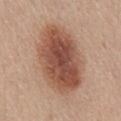Part of a total-body skin-imaging series; this lesion was reviewed on a skin check and was not flagged for biopsy. Located on the lower back. Automated tile analysis of the lesion measured a footprint of about 38 mm², a shape eccentricity near 0.8, and two-axis asymmetry of about 0.1. It also reported a mean CIELAB color near L≈51 a*≈22 b*≈29, roughly 15 lightness units darker than nearby skin, and a normalized border contrast of about 10. A 15 mm close-up tile from a total-body photography series done for melanoma screening. The patient is a female aged 53 to 57. Approximately 9 mm at its widest.